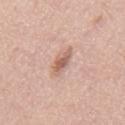<tbp_lesion>
<biopsy_status>not biopsied; imaged during a skin examination</biopsy_status>
<lesion_size>
  <long_diameter_mm_approx>3.5</long_diameter_mm_approx>
</lesion_size>
<image>
  <source>total-body photography crop</source>
  <field_of_view_mm>15</field_of_view_mm>
</image>
<lighting>white-light</lighting>
<site>mid back</site>
<patient>
  <sex>male</sex>
  <age_approx>45</age_approx>
</patient>
<automated_metrics>
  <area_mm2_approx>4.5</area_mm2_approx>
  <shape_asymmetry>0.3</shape_asymmetry>
  <cielab_L>61</cielab_L>
  <cielab_a>21</cielab_a>
  <cielab_b>27</cielab_b>
  <vs_skin_darker_L>12.0</vs_skin_darker_L>
  <vs_skin_contrast_norm>7.5</vs_skin_contrast_norm>
  <nevus_likeness_0_100>60</nevus_likeness_0_100>
  <lesion_detection_confidence_0_100>100</lesion_detection_confidence_0_100>
</automated_metrics>
</tbp_lesion>